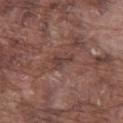Case summary:
• notes · catalogued during a skin exam; not biopsied
• TBP lesion metrics · a within-lesion color-variation index near 1/10 and a peripheral color-asymmetry measure near 0.5; a classifier nevus-likeness of about 0/100 and a detector confidence of about 85 out of 100 that the crop contains a lesion
• size · ≈3 mm
• site · the left thigh
• imaging modality · 15 mm crop, total-body photography
• patient · male, aged 73 to 77